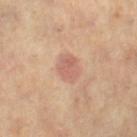The lesion was photographed on a routine skin check and not biopsied; there is no pathology result. Imaged with cross-polarized lighting. Automated image analysis of the tile measured an outline eccentricity of about 0.6 (0 = round, 1 = elongated) and a shape-asymmetry score of about 0.15 (0 = symmetric). The software also gave a mean CIELAB color near L≈60 a*≈22 b*≈28, roughly 9 lightness units darker than nearby skin, and a normalized lesion–skin contrast near 6. The analysis additionally found a border-irregularity index near 1.5/10 and a color-variation rating of about 2.5/10. The analysis additionally found a nevus-likeness score of about 20/100 and lesion-presence confidence of about 100/100. A female subject roughly 40 years of age. A region of skin cropped from a whole-body photographic capture, roughly 15 mm wide. Approximately 3 mm at its widest. Located on the leg.